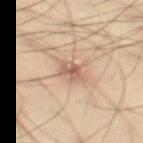The lesion was tiled from a total-body skin photograph and was not biopsied. The lesion is located on the right thigh. The subject is a male aged around 40. A region of skin cropped from a whole-body photographic capture, roughly 15 mm wide.